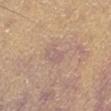Case summary:
- workup — catalogued during a skin exam; not biopsied
- image source — ~15 mm tile from a whole-body skin photo
- site — the left thigh
- tile lighting — white-light
- lesion size — ≈3 mm
- patient — male, aged approximately 65
- TBP lesion metrics — a border-irregularity index near 2.5/10, a within-lesion color-variation index near 2/10, and a peripheral color-asymmetry measure near 0.5